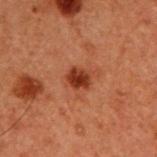notes: total-body-photography surveillance lesion; no biopsy | tile lighting: cross-polarized illumination | image source: 15 mm crop, total-body photography | size: about 2.5 mm | location: the upper back | automated metrics: a normalized lesion–skin contrast near 10; internal color variation of about 2.5 on a 0–10 scale and radial color variation of about 1; an automated nevus-likeness rating near 95 out of 100 and lesion-presence confidence of about 100/100 | subject: male, aged around 60.